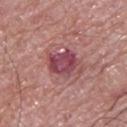This lesion was catalogued during total-body skin photography and was not selected for biopsy.
About 4 mm across.
Imaged with white-light lighting.
A lesion tile, about 15 mm wide, cut from a 3D total-body photograph.
From the upper back.
A male subject, aged around 65.